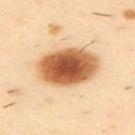follow-up: total-body-photography surveillance lesion; no biopsy | diameter: ≈7 mm | subject: male, in their 40s | image: ~15 mm crop, total-body skin-cancer survey | lighting: cross-polarized | body site: the upper back.